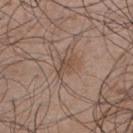Imaged during a routine full-body skin examination; the lesion was not biopsied and no histopathology is available. A male subject in their 50s. Captured under white-light illumination. The lesion is located on the upper back. A 15 mm close-up extracted from a 3D total-body photography capture. The total-body-photography lesion software estimated a border-irregularity index near 4/10, a within-lesion color-variation index near 2.5/10, and radial color variation of about 1. Approximately 3 mm at its widest.